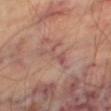Assessment: This lesion was catalogued during total-body skin photography and was not selected for biopsy. Background: From the right thigh. The patient is a male roughly 70 years of age. A 15 mm crop from a total-body photograph taken for skin-cancer surveillance.